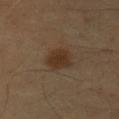notes=imaged on a skin check; not biopsied
subject=male, approximately 55 years of age
image source=total-body-photography crop, ~15 mm field of view
size=about 3.5 mm
anatomic site=the chest
lighting=cross-polarized illumination